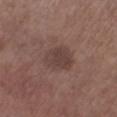Q: Is there a histopathology result?
A: catalogued during a skin exam; not biopsied
Q: What did automated image analysis measure?
A: about 8 CIELAB-L* units darker than the surrounding skin and a normalized border contrast of about 6.5; border irregularity of about 1.5 on a 0–10 scale and a peripheral color-asymmetry measure near 1
Q: What is the lesion's diameter?
A: about 4 mm
Q: What lighting was used for the tile?
A: white-light
Q: What is the imaging modality?
A: 15 mm crop, total-body photography
Q: Who is the patient?
A: male, roughly 55 years of age
Q: Where on the body is the lesion?
A: the right lower leg